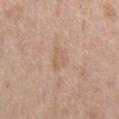follow-up: catalogued during a skin exam; not biopsied | location: the chest | image source: total-body-photography crop, ~15 mm field of view | subject: male, roughly 55 years of age | image-analysis metrics: an area of roughly 4 mm², an eccentricity of roughly 0.8, and a shape-asymmetry score of about 0.3 (0 = symmetric) | tile lighting: white-light.